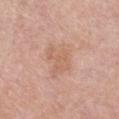workup: imaged on a skin check; not biopsied
patient: female, roughly 40 years of age
anatomic site: the chest
image: total-body-photography crop, ~15 mm field of view